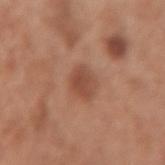Case summary:
- workup — no biopsy performed (imaged during a skin exam)
- body site — the left forearm
- automated metrics — a mean CIELAB color near L≈48 a*≈24 b*≈30, roughly 9 lightness units darker than nearby skin, and a normalized lesion–skin contrast near 7; a color-variation rating of about 2/10 and a peripheral color-asymmetry measure near 0.5
- subject — female, about 50 years old
- image — 15 mm crop, total-body photography
- lesion diameter — ≈2.5 mm